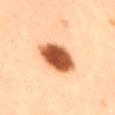Imaged during a routine full-body skin examination; the lesion was not biopsied and no histopathology is available.
The total-body-photography lesion software estimated a border-irregularity rating of about 1.5/10 and a within-lesion color-variation index near 6/10. The software also gave an automated nevus-likeness rating near 100 out of 100 and a detector confidence of about 100 out of 100 that the crop contains a lesion.
Imaged with cross-polarized lighting.
A male subject, aged around 50.
On the right thigh.
A lesion tile, about 15 mm wide, cut from a 3D total-body photograph.
The recorded lesion diameter is about 4.5 mm.